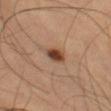{
  "biopsy_status": "not biopsied; imaged during a skin examination",
  "lighting": "cross-polarized",
  "image": {
    "source": "total-body photography crop",
    "field_of_view_mm": 15
  },
  "patient": {
    "sex": "male",
    "age_approx": 60
  },
  "site": "left thigh"
}